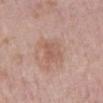Impression:
No biopsy was performed on this lesion — it was imaged during a full skin examination and was not determined to be concerning.
Background:
Located on the mid back. Captured under white-light illumination. A male patient aged around 75. A 15 mm close-up tile from a total-body photography series done for melanoma screening. About 4 mm across.